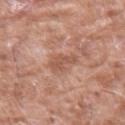Q: Is there a histopathology result?
A: no biopsy performed (imaged during a skin exam)
Q: Patient demographics?
A: male, in their 60s
Q: How large is the lesion?
A: about 3 mm
Q: What kind of image is this?
A: 15 mm crop, total-body photography
Q: Where on the body is the lesion?
A: the left forearm
Q: Automated lesion metrics?
A: a lesion area of about 4.5 mm², a shape eccentricity near 0.8, and two-axis asymmetry of about 0.4; a mean CIELAB color near L≈54 a*≈23 b*≈30, a lesion–skin lightness drop of about 8, and a normalized lesion–skin contrast near 6